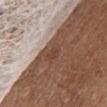<case>
<biopsy_status>not biopsied; imaged during a skin examination</biopsy_status>
<automated_metrics>
  <nevus_likeness_0_100>0</nevus_likeness_0_100>
  <lesion_detection_confidence_0_100>55</lesion_detection_confidence_0_100>
</automated_metrics>
<lesion_size>
  <long_diameter_mm_approx>3.0</long_diameter_mm_approx>
</lesion_size>
<site>head or neck</site>
<patient>
  <sex>female</sex>
  <age_approx>70</age_approx>
</patient>
<image>
  <source>total-body photography crop</source>
  <field_of_view_mm>15</field_of_view_mm>
</image>
<lighting>white-light</lighting>
</case>